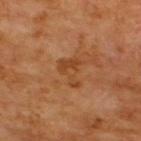An algorithmic analysis of the crop reported an area of roughly 5.5 mm², an eccentricity of roughly 0.75, and a shape-asymmetry score of about 0.65 (0 = symmetric). And it measured a lesion color around L≈43 a*≈24 b*≈38 in CIELAB and a normalized lesion–skin contrast near 6. The software also gave a border-irregularity rating of about 7/10, internal color variation of about 2.5 on a 0–10 scale, and peripheral color asymmetry of about 0.5. The software also gave an automated nevus-likeness rating near 0 out of 100.
From the upper back.
Longest diameter approximately 3.5 mm.
A male subject aged 63–67.
Imaged with cross-polarized lighting.
A 15 mm close-up tile from a total-body photography series done for melanoma screening.